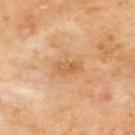The lesion was photographed on a routine skin check and not biopsied; there is no pathology result. From the upper back. Cropped from a total-body skin-imaging series; the visible field is about 15 mm. Automated tile analysis of the lesion measured a lesion–skin lightness drop of about 8 and a normalized lesion–skin contrast near 6. The analysis additionally found a border-irregularity index near 3.5/10, internal color variation of about 3.5 on a 0–10 scale, and peripheral color asymmetry of about 1.5. Captured under cross-polarized illumination.